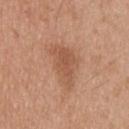Imaged during a routine full-body skin examination; the lesion was not biopsied and no histopathology is available. Measured at roughly 5.5 mm in maximum diameter. This image is a 15 mm lesion crop taken from a total-body photograph. A male patient aged 33 to 37. From the head or neck. Imaged with white-light lighting.